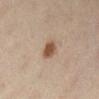Captured during whole-body skin photography for melanoma surveillance; the lesion was not biopsied.
A female patient, in their mid- to late 40s.
The lesion is located on the left lower leg.
A lesion tile, about 15 mm wide, cut from a 3D total-body photograph.